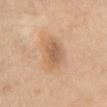Findings:
• imaging modality · ~15 mm tile from a whole-body skin photo
• site · the chest
• patient · female, aged 68 to 72